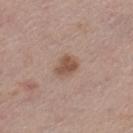biopsy status: total-body-photography surveillance lesion; no biopsy
patient: female, in their mid-50s
acquisition: ~15 mm tile from a whole-body skin photo
size: ~3 mm (longest diameter)
site: the left thigh